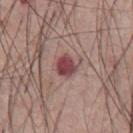Findings:
– workup — imaged on a skin check; not biopsied
– lighting — white-light illumination
– acquisition — 15 mm crop, total-body photography
– subject — male, aged 53–57
– site — the abdomen